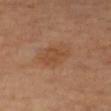follow-up=imaged on a skin check; not biopsied | subject=female, roughly 65 years of age | acquisition=~15 mm crop, total-body skin-cancer survey | body site=the right forearm.